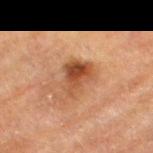  biopsy_status: not biopsied; imaged during a skin examination
  image:
    source: total-body photography crop
    field_of_view_mm: 15
  lesion_size:
    long_diameter_mm_approx: 4.0
  lighting: cross-polarized
  patient:
    sex: male
    age_approx: 85+
  site: left thigh